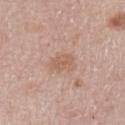follow-up: catalogued during a skin exam; not biopsied
subject: female, in their 50s
diameter: about 2.5 mm
automated lesion analysis: a lesion area of about 4.5 mm², a shape eccentricity near 0.65, and two-axis asymmetry of about 0.2; a classifier nevus-likeness of about 0/100 and lesion-presence confidence of about 100/100
anatomic site: the left upper arm
acquisition: 15 mm crop, total-body photography